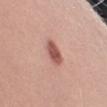The lesion is located on the left upper arm.
The total-body-photography lesion software estimated an outline eccentricity of about 0.7 (0 = round, 1 = elongated) and two-axis asymmetry of about 0.1. And it measured a border-irregularity index near 1.5/10 and radial color variation of about 1. The software also gave lesion-presence confidence of about 100/100.
Longest diameter approximately 3 mm.
Captured under white-light illumination.
A close-up tile cropped from a whole-body skin photograph, about 15 mm across.
A male subject aged around 25.